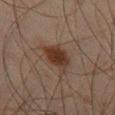Q: Was this lesion biopsied?
A: imaged on a skin check; not biopsied
Q: What is the imaging modality?
A: 15 mm crop, total-body photography
Q: Patient demographics?
A: male, roughly 45 years of age
Q: Illumination type?
A: cross-polarized illumination
Q: Lesion location?
A: the right thigh